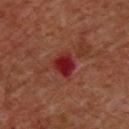Imaged during a routine full-body skin examination; the lesion was not biopsied and no histopathology is available. A roughly 15 mm field-of-view crop from a total-body skin photograph. A male patient, aged around 60. From the chest.